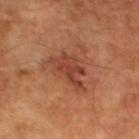Assessment: This lesion was catalogued during total-body skin photography and was not selected for biopsy. Acquisition and patient details: This is a cross-polarized tile. A male patient, aged 63 to 67. About 5 mm across. The lesion-visualizer software estimated a lesion area of about 11 mm² and a shape eccentricity near 0.75. And it measured about 9 CIELAB-L* units darker than the surrounding skin and a normalized lesion–skin contrast near 7.5. The analysis additionally found a classifier nevus-likeness of about 15/100. A 15 mm close-up tile from a total-body photography series done for melanoma screening.